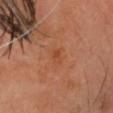| key | value |
|---|---|
| notes | catalogued during a skin exam; not biopsied |
| diameter | about 2.5 mm |
| body site | the head or neck |
| acquisition | ~15 mm crop, total-body skin-cancer survey |